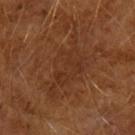Automated image analysis of the tile measured a lesion area of about 15 mm² and a shape eccentricity near 0.85. And it measured a mean CIELAB color near L≈32 a*≈21 b*≈30 and a lesion–skin lightness drop of about 5. It also reported border irregularity of about 7.5 on a 0–10 scale, a within-lesion color-variation index near 2.5/10, and peripheral color asymmetry of about 1.
The lesion's longest dimension is about 7.5 mm.
A roughly 15 mm field-of-view crop from a total-body skin photograph.
A male patient, aged approximately 65.
The tile uses cross-polarized illumination.